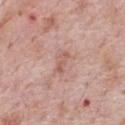biopsy status = imaged on a skin check; not biopsied
acquisition = ~15 mm tile from a whole-body skin photo
patient = male, about 70 years old
lesion diameter = ~3 mm (longest diameter)
image-analysis metrics = a lesion area of about 3 mm², a shape eccentricity near 0.9, and a symmetry-axis asymmetry near 0.3; a mean CIELAB color near L≈58 a*≈23 b*≈27, a lesion–skin lightness drop of about 8, and a normalized border contrast of about 5.5; a border-irregularity index near 4/10, a color-variation rating of about 0/10, and a peripheral color-asymmetry measure near 0; a classifier nevus-likeness of about 0/100 and a detector confidence of about 100 out of 100 that the crop contains a lesion
location = the chest